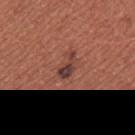Notes:
• workup: no biopsy performed (imaged during a skin exam)
• patient: female, roughly 35 years of age
• location: the right upper arm
• size: ≈3 mm
• image: 15 mm crop, total-body photography